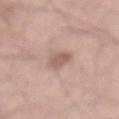The lesion was tiled from a total-body skin photograph and was not biopsied. A male subject, in their 70s. The recorded lesion diameter is about 3.5 mm. A close-up tile cropped from a whole-body skin photograph, about 15 mm across. From the mid back.